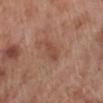Clinical impression: Part of a total-body skin-imaging series; this lesion was reviewed on a skin check and was not flagged for biopsy. Clinical summary: This image is a 15 mm lesion crop taken from a total-body photograph. The tile uses white-light illumination. An algorithmic analysis of the crop reported border irregularity of about 2.5 on a 0–10 scale, internal color variation of about 1 on a 0–10 scale, and a peripheral color-asymmetry measure near 0. The lesion's longest dimension is about 3 mm. A male patient aged around 70. On the left lower leg.